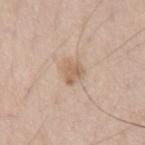Clinical impression: Part of a total-body skin-imaging series; this lesion was reviewed on a skin check and was not flagged for biopsy. Background: Located on the chest. A 15 mm close-up extracted from a 3D total-body photography capture. Automated image analysis of the tile measured border irregularity of about 3.5 on a 0–10 scale and peripheral color asymmetry of about 1. A male patient, aged 68 to 72.